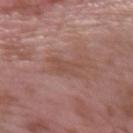Case summary:
* patient · male, aged around 40
* diameter · about 4 mm
* image source · ~15 mm tile from a whole-body skin photo
* site · the left forearm
* lighting · white-light illumination